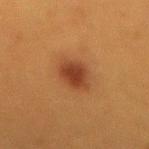The lesion was tiled from a total-body skin photograph and was not biopsied. Longest diameter approximately 4.5 mm. The total-body-photography lesion software estimated an eccentricity of roughly 0.75 and two-axis asymmetry of about 0.15. And it measured an average lesion color of about L≈35 a*≈22 b*≈30 (CIELAB), roughly 9 lightness units darker than nearby skin, and a normalized lesion–skin contrast near 8.5. The software also gave a border-irregularity rating of about 1.5/10, a within-lesion color-variation index near 3.5/10, and peripheral color asymmetry of about 1. A female patient, about 40 years old. From the mid back. A region of skin cropped from a whole-body photographic capture, roughly 15 mm wide.